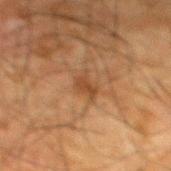Imaged during a routine full-body skin examination; the lesion was not biopsied and no histopathology is available.
From the mid back.
The lesion's longest dimension is about 2.5 mm.
The subject is a male in their mid- to late 60s.
A 15 mm close-up extracted from a 3D total-body photography capture.
Imaged with cross-polarized lighting.